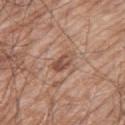biopsy status: catalogued during a skin exam; not biopsied | acquisition: total-body-photography crop, ~15 mm field of view | subject: male, about 60 years old | automated metrics: an area of roughly 4.5 mm² and an eccentricity of roughly 0.8; an automated nevus-likeness rating near 10 out of 100 | lesion size: about 3 mm | tile lighting: white-light | location: the right thigh.